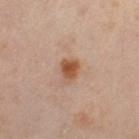| field | value |
|---|---|
| workup | no biopsy performed (imaged during a skin exam) |
| site | the left thigh |
| size | about 2.5 mm |
| image | ~15 mm crop, total-body skin-cancer survey |
| tile lighting | cross-polarized |
| patient | female, aged approximately 40 |